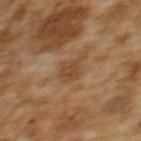workup: total-body-photography surveillance lesion; no biopsy | tile lighting: cross-polarized | patient: female, approximately 60 years of age | image-analysis metrics: a footprint of about 5 mm²; a lesion–skin lightness drop of about 6 | image: ~15 mm crop, total-body skin-cancer survey | site: the upper back | lesion size: ≈2.5 mm.